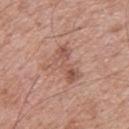Assessment: This lesion was catalogued during total-body skin photography and was not selected for biopsy. Background: On the upper back. The patient is a male approximately 70 years of age. A roughly 15 mm field-of-view crop from a total-body skin photograph.